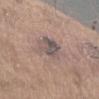  biopsy_status: not biopsied; imaged during a skin examination
  site: left forearm
  lighting: white-light
  patient:
    sex: male
    age_approx: 70
  image:
    source: total-body photography crop
    field_of_view_mm: 15
  lesion_size:
    long_diameter_mm_approx: 4.5